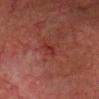The lesion was tiled from a total-body skin photograph and was not biopsied. Cropped from a total-body skin-imaging series; the visible field is about 15 mm. A male subject, about 75 years old. The lesion is on the head or neck.